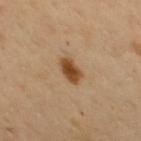The lesion was photographed on a routine skin check and not biopsied; there is no pathology result. A 15 mm close-up extracted from a 3D total-body photography capture. This is a cross-polarized tile. The lesion is located on the chest. A male patient about 50 years old.